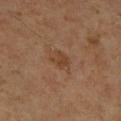notes: no biopsy performed (imaged during a skin exam)
image-analysis metrics: a mean CIELAB color near L≈41 a*≈18 b*≈31, a lesion–skin lightness drop of about 6, and a normalized lesion–skin contrast near 6; a border-irregularity index near 2/10, a color-variation rating of about 3/10, and radial color variation of about 1; lesion-presence confidence of about 100/100
imaging modality: 15 mm crop, total-body photography
anatomic site: the right lower leg
patient: female, about 65 years old
illumination: cross-polarized illumination